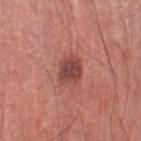Q: Lesion location?
A: the right thigh
Q: What is the imaging modality?
A: 15 mm crop, total-body photography
Q: Automated lesion metrics?
A: an outline eccentricity of about 0.4 (0 = round, 1 = elongated) and a shape-asymmetry score of about 0.2 (0 = symmetric); an automated nevus-likeness rating near 60 out of 100 and a detector confidence of about 100 out of 100 that the crop contains a lesion
Q: How large is the lesion?
A: ≈3 mm
Q: Who is the patient?
A: male, roughly 70 years of age